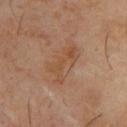The lesion was photographed on a routine skin check and not biopsied; there is no pathology result.
The tile uses cross-polarized illumination.
The patient is a male about 65 years old.
Approximately 4.5 mm at its widest.
An algorithmic analysis of the crop reported a border-irregularity rating of about 4/10, internal color variation of about 4 on a 0–10 scale, and radial color variation of about 1. The software also gave an automated nevus-likeness rating near 0 out of 100 and a detector confidence of about 100 out of 100 that the crop contains a lesion.
Located on the upper back.
Cropped from a whole-body photographic skin survey; the tile spans about 15 mm.